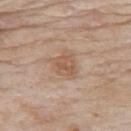Imaged during a routine full-body skin examination; the lesion was not biopsied and no histopathology is available. The subject is a male roughly 85 years of age or older. The lesion is located on the upper back. Imaged with white-light lighting. The lesion's longest dimension is about 3.5 mm. A 15 mm close-up tile from a total-body photography series done for melanoma screening.